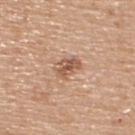<record>
<biopsy_status>not biopsied; imaged during a skin examination</biopsy_status>
<lesion_size>
  <long_diameter_mm_approx>3.0</long_diameter_mm_approx>
</lesion_size>
<patient>
  <sex>male</sex>
  <age_approx>55</age_approx>
</patient>
<image>
  <source>total-body photography crop</source>
  <field_of_view_mm>15</field_of_view_mm>
</image>
<site>back</site>
</record>